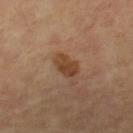Impression:
Imaged during a routine full-body skin examination; the lesion was not biopsied and no histopathology is available.
Context:
The subject is a female approximately 65 years of age. The tile uses cross-polarized illumination. Approximately 3.5 mm at its widest. A close-up tile cropped from a whole-body skin photograph, about 15 mm across. From the left thigh.